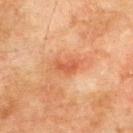Clinical impression:
This lesion was catalogued during total-body skin photography and was not selected for biopsy.
Clinical summary:
About 3 mm across. A male subject approximately 75 years of age. A close-up tile cropped from a whole-body skin photograph, about 15 mm across. This is a cross-polarized tile. The lesion is on the upper back.